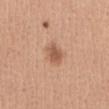<lesion>
  <biopsy_status>not biopsied; imaged during a skin examination</biopsy_status>
  <site>abdomen</site>
  <image>
    <source>total-body photography crop</source>
    <field_of_view_mm>15</field_of_view_mm>
  </image>
  <lighting>white-light</lighting>
  <lesion_size>
    <long_diameter_mm_approx>2.5</long_diameter_mm_approx>
  </lesion_size>
  <patient>
    <sex>female</sex>
    <age_approx>40</age_approx>
  </patient>
  <automated_metrics>
    <area_mm2_approx>5.0</area_mm2_approx>
    <eccentricity>0.5</eccentricity>
    <shape_asymmetry>0.25</shape_asymmetry>
    <color_variation_0_10>2.5</color_variation_0_10>
    <peripheral_color_asymmetry>1.0</peripheral_color_asymmetry>
  </automated_metrics>
</lesion>